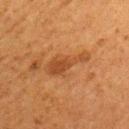Notes:
– workup: imaged on a skin check; not biopsied
– lighting: cross-polarized illumination
– image source: total-body-photography crop, ~15 mm field of view
– subject: female, aged around 50
– site: the left upper arm
– lesion size: about 5.5 mm